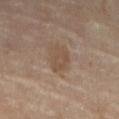Recorded during total-body skin imaging; not selected for excision or biopsy.
The total-body-photography lesion software estimated a footprint of about 5.5 mm², an eccentricity of roughly 0.75, and a shape-asymmetry score of about 0.3 (0 = symmetric). And it measured a border-irregularity index near 3.5/10, a within-lesion color-variation index near 2/10, and peripheral color asymmetry of about 0.5. The analysis additionally found a classifier nevus-likeness of about 5/100 and a lesion-detection confidence of about 100/100.
Imaged with cross-polarized lighting.
Located on the leg.
The patient is a female roughly 65 years of age.
A roughly 15 mm field-of-view crop from a total-body skin photograph.